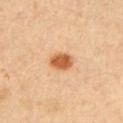<record>
  <biopsy_status>not biopsied; imaged during a skin examination</biopsy_status>
  <patient>
    <sex>male</sex>
    <age_approx>55</age_approx>
  </patient>
  <image>
    <source>total-body photography crop</source>
    <field_of_view_mm>15</field_of_view_mm>
  </image>
  <site>left upper arm</site>
  <lesion_size>
    <long_diameter_mm_approx>3.0</long_diameter_mm_approx>
  </lesion_size>
  <lighting>cross-polarized</lighting>
  <automated_metrics>
    <area_mm2_approx>6.0</area_mm2_approx>
    <shape_asymmetry>0.15</shape_asymmetry>
    <cielab_L>58</cielab_L>
    <cielab_a>26</cielab_a>
    <cielab_b>40</cielab_b>
    <vs_skin_darker_L>15.0</vs_skin_darker_L>
    <vs_skin_contrast_norm>10.0</vs_skin_contrast_norm>
    <nevus_likeness_0_100>100</nevus_likeness_0_100>
    <lesion_detection_confidence_0_100>100</lesion_detection_confidence_0_100>
  </automated_metrics>
</record>